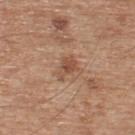Q: Was a biopsy performed?
A: total-body-photography surveillance lesion; no biopsy
Q: Lesion location?
A: the upper back
Q: How was this image acquired?
A: 15 mm crop, total-body photography
Q: What is the lesion's diameter?
A: ~3.5 mm (longest diameter)
Q: Who is the patient?
A: male, in their mid-60s
Q: Illumination type?
A: white-light illumination